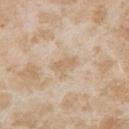Captured during whole-body skin photography for melanoma surveillance; the lesion was not biopsied.
A female subject, aged approximately 25.
This image is a 15 mm lesion crop taken from a total-body photograph.
An algorithmic analysis of the crop reported an area of roughly 3.5 mm², an eccentricity of roughly 0.85, and a shape-asymmetry score of about 0.35 (0 = symmetric). And it measured a lesion color around L≈65 a*≈14 b*≈33 in CIELAB and about 8 CIELAB-L* units darker than the surrounding skin.
From the right upper arm.